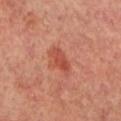Notes:
- workup: imaged on a skin check; not biopsied
- image source: ~15 mm tile from a whole-body skin photo
- anatomic site: the left lower leg
- image-analysis metrics: border irregularity of about 2.5 on a 0–10 scale; a classifier nevus-likeness of about 70/100 and a detector confidence of about 100 out of 100 that the crop contains a lesion
- lighting: cross-polarized
- size: about 3.5 mm
- patient: male, aged around 65